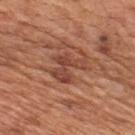No biopsy was performed on this lesion — it was imaged during a full skin examination and was not determined to be concerning. The patient is a male roughly 65 years of age. This is a white-light tile. Cropped from a whole-body photographic skin survey; the tile spans about 15 mm. The lesion is on the upper back.